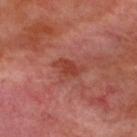{"biopsy_status": "not biopsied; imaged during a skin examination", "lesion_size": {"long_diameter_mm_approx": 3.5}, "automated_metrics": {"nevus_likeness_0_100": 10, "lesion_detection_confidence_0_100": 100}, "patient": {"sex": "male", "age_approx": 50}, "lighting": "cross-polarized", "site": "upper back", "image": {"source": "total-body photography crop", "field_of_view_mm": 15}}